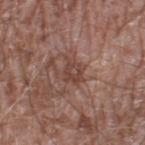workup: no biopsy performed (imaged during a skin exam); imaging modality: total-body-photography crop, ~15 mm field of view; illumination: white-light illumination; site: the leg; lesion diameter: ≈3.5 mm; patient: male, aged 58 to 62.